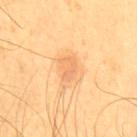biopsy_status: not biopsied; imaged during a skin examination
site: chest
image:
  source: total-body photography crop
  field_of_view_mm: 15
automated_metrics:
  eccentricity: 0.75
  shape_asymmetry: 0.25
  vs_skin_darker_L: 8.0
  border_irregularity_0_10: 2.5
  color_variation_0_10: 2.0
  peripheral_color_asymmetry: 1.0
  nevus_likeness_0_100: 20
patient:
  sex: male
  age_approx: 60